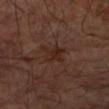The lesion was tiled from a total-body skin photograph and was not biopsied. On the left forearm. A 15 mm close-up tile from a total-body photography series done for melanoma screening. A male patient roughly 65 years of age. The total-body-photography lesion software estimated a lesion color around L≈23 a*≈19 b*≈22 in CIELAB, a lesion–skin lightness drop of about 6, and a normalized lesion–skin contrast near 7. And it measured a classifier nevus-likeness of about 5/100 and a detector confidence of about 100 out of 100 that the crop contains a lesion. Captured under cross-polarized illumination. About 2.5 mm across.